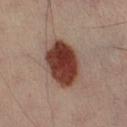• notes: no biopsy performed (imaged during a skin exam)
• subject: male, aged 48–52
• anatomic site: the right lower leg
• image source: 15 mm crop, total-body photography
• lighting: cross-polarized